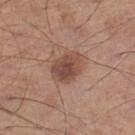Imaged during a routine full-body skin examination; the lesion was not biopsied and no histopathology is available.
The tile uses white-light illumination.
Automated image analysis of the tile measured a shape-asymmetry score of about 0.15 (0 = symmetric). It also reported a nevus-likeness score of about 65/100 and a lesion-detection confidence of about 100/100.
The lesion is located on the left lower leg.
A 15 mm close-up tile from a total-body photography series done for melanoma screening.
The subject is a male aged approximately 60.